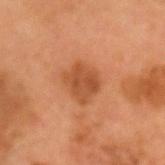This lesion was catalogued during total-body skin photography and was not selected for biopsy. The lesion is located on the head or neck. The lesion-visualizer software estimated a color-variation rating of about 2.5/10 and radial color variation of about 1. And it measured a nevus-likeness score of about 45/100. Imaged with cross-polarized lighting. About 4 mm across. A roughly 15 mm field-of-view crop from a total-body skin photograph. A male subject, in their mid- to late 50s.